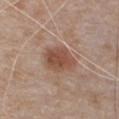| key | value |
|---|---|
| workup | catalogued during a skin exam; not biopsied |
| patient | male, aged 78–82 |
| anatomic site | the chest |
| acquisition | ~15 mm tile from a whole-body skin photo |